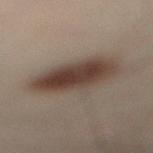Captured during whole-body skin photography for melanoma surveillance; the lesion was not biopsied. A male patient, aged 48 to 52. The lesion is on the left leg. Imaged with cross-polarized lighting. A lesion tile, about 15 mm wide, cut from a 3D total-body photograph. About 6 mm across. An algorithmic analysis of the crop reported an area of roughly 23 mm², a shape eccentricity near 0.65, and two-axis asymmetry of about 0.1. The analysis additionally found a lesion color around L≈32 a*≈12 b*≈19 in CIELAB, a lesion–skin lightness drop of about 11, and a normalized lesion–skin contrast near 10. The analysis additionally found a border-irregularity rating of about 1.5/10 and internal color variation of about 5 on a 0–10 scale.